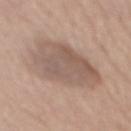Q: Was a biopsy performed?
A: total-body-photography surveillance lesion; no biopsy
Q: What kind of image is this?
A: ~15 mm tile from a whole-body skin photo
Q: Patient demographics?
A: male, in their 60s
Q: What lighting was used for the tile?
A: white-light
Q: What did automated image analysis measure?
A: a mean CIELAB color near L≈56 a*≈16 b*≈24 and a lesion-to-skin contrast of about 7 (normalized; higher = more distinct); a nevus-likeness score of about 0/100 and a lesion-detection confidence of about 100/100
Q: Lesion location?
A: the back
Q: What is the lesion's diameter?
A: ≈9 mm